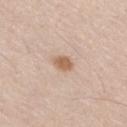Clinical impression:
Recorded during total-body skin imaging; not selected for excision or biopsy.
Acquisition and patient details:
Captured under white-light illumination. A male subject, approximately 60 years of age. This image is a 15 mm lesion crop taken from a total-body photograph. The lesion is on the right thigh. The lesion's longest dimension is about 2.5 mm.